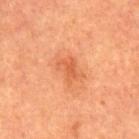The lesion was photographed on a routine skin check and not biopsied; there is no pathology result.
The tile uses cross-polarized illumination.
The subject is a male aged around 65.
About 3 mm across.
On the abdomen.
Automated tile analysis of the lesion measured a lesion–skin lightness drop of about 8 and a normalized lesion–skin contrast near 5.5. The analysis additionally found border irregularity of about 4.5 on a 0–10 scale, a within-lesion color-variation index near 1.5/10, and a peripheral color-asymmetry measure near 0.5. It also reported an automated nevus-likeness rating near 10 out of 100.
A roughly 15 mm field-of-view crop from a total-body skin photograph.